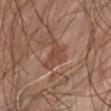The lesion was tiled from a total-body skin photograph and was not biopsied.
A 15 mm crop from a total-body photograph taken for skin-cancer surveillance.
About 4 mm across.
From the abdomen.
A male patient aged approximately 60.
The lesion-visualizer software estimated an area of roughly 6.5 mm² and a shape eccentricity near 0.8. The analysis additionally found a lesion color around L≈41 a*≈20 b*≈25 in CIELAB. The software also gave a border-irregularity index near 6.5/10, a color-variation rating of about 3/10, and radial color variation of about 1. The software also gave a classifier nevus-likeness of about 0/100 and lesion-presence confidence of about 75/100.